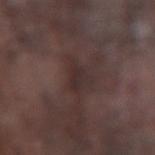Q: Was this lesion biopsied?
A: imaged on a skin check; not biopsied
Q: What is the anatomic site?
A: the left forearm
Q: What is the imaging modality?
A: ~15 mm crop, total-body skin-cancer survey
Q: What lighting was used for the tile?
A: white-light illumination
Q: Who is the patient?
A: male, aged 73 to 77